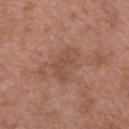| feature | finding |
|---|---|
| notes | total-body-photography surveillance lesion; no biopsy |
| tile lighting | white-light |
| image | ~15 mm tile from a whole-body skin photo |
| patient | male, aged approximately 50 |
| lesion diameter | ~4 mm (longest diameter) |
| TBP lesion metrics | a lesion area of about 8 mm², an outline eccentricity of about 0.75 (0 = round, 1 = elongated), and a shape-asymmetry score of about 0.35 (0 = symmetric); a mean CIELAB color near L≈49 a*≈21 b*≈28, a lesion–skin lightness drop of about 6, and a lesion-to-skin contrast of about 5 (normalized; higher = more distinct); a nevus-likeness score of about 0/100 and a lesion-detection confidence of about 100/100 |
| site | the head or neck |